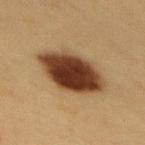Findings:
– biopsy status — imaged on a skin check; not biopsied
– lighting — cross-polarized illumination
– imaging modality — 15 mm crop, total-body photography
– subject — female, in their 40s
– site — the mid back
– lesion diameter — ~7 mm (longest diameter)